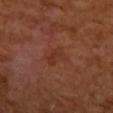Q: Is there a histopathology result?
A: imaged on a skin check; not biopsied
Q: Illumination type?
A: cross-polarized illumination
Q: What did automated image analysis measure?
A: roughly 5 lightness units darker than nearby skin and a normalized lesion–skin contrast near 5.5; a border-irregularity index near 4/10 and a peripheral color-asymmetry measure near 0.5; a nevus-likeness score of about 0/100
Q: Lesion location?
A: the right upper arm
Q: Patient demographics?
A: female, aged 48 to 52
Q: What kind of image is this?
A: 15 mm crop, total-body photography